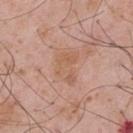Image and clinical context: A 15 mm close-up tile from a total-body photography series done for melanoma screening. A male patient about 55 years old. The lesion is on the upper back. Automated tile analysis of the lesion measured a mean CIELAB color near L≈58 a*≈21 b*≈31 and a lesion-to-skin contrast of about 5 (normalized; higher = more distinct). And it measured a border-irregularity index near 5/10, internal color variation of about 3 on a 0–10 scale, and radial color variation of about 1. And it measured a classifier nevus-likeness of about 0/100 and lesion-presence confidence of about 100/100. This is a white-light tile.